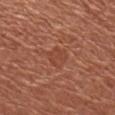{"biopsy_status": "not biopsied; imaged during a skin examination", "site": "arm", "image": {"source": "total-body photography crop", "field_of_view_mm": 15}, "patient": {"sex": "female", "age_approx": 65}}